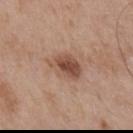Recorded during total-body skin imaging; not selected for excision or biopsy. Located on the chest. A male subject, approximately 65 years of age. This image is a 15 mm lesion crop taken from a total-body photograph. Longest diameter approximately 4 mm.